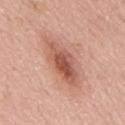Notes:
– location · the back
– image source · 15 mm crop, total-body photography
– lesion diameter · ≈7 mm
– patient · male, about 65 years old
– illumination · white-light